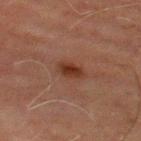No biopsy was performed on this lesion — it was imaged during a full skin examination and was not determined to be concerning. The lesion is on the leg. A male subject, aged around 70. A 15 mm close-up tile from a total-body photography series done for melanoma screening. Measured at roughly 3 mm in maximum diameter.